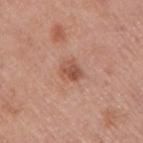Image and clinical context: Cropped from a total-body skin-imaging series; the visible field is about 15 mm. Captured under white-light illumination. The lesion-visualizer software estimated a lesion area of about 4.5 mm², an outline eccentricity of about 0.65 (0 = round, 1 = elongated), and a shape-asymmetry score of about 0.25 (0 = symmetric). The analysis additionally found an average lesion color of about L≈53 a*≈24 b*≈30 (CIELAB) and a normalized border contrast of about 7. A male patient, aged approximately 55. From the right upper arm.